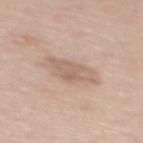| key | value |
|---|---|
| follow-up | imaged on a skin check; not biopsied |